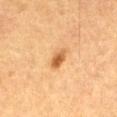follow-up: no biopsy performed (imaged during a skin exam) | lighting: cross-polarized illumination | subject: male, aged around 85 or older | diameter: about 2.5 mm | location: the front of the torso | imaging modality: ~15 mm crop, total-body skin-cancer survey | automated metrics: a border-irregularity rating of about 1.5/10, a color-variation rating of about 3.5/10, and radial color variation of about 1.